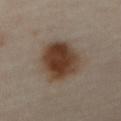Impression: The lesion was tiled from a total-body skin photograph and was not biopsied. Clinical summary: The lesion is located on the abdomen. A male subject, in their mid- to late 60s. Cropped from a whole-body photographic skin survey; the tile spans about 15 mm.